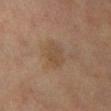<record>
<lighting>cross-polarized</lighting>
<site>right lower leg</site>
<lesion_size>
  <long_diameter_mm_approx>3.0</long_diameter_mm_approx>
</lesion_size>
<patient>
  <sex>female</sex>
  <age_approx>70</age_approx>
</patient>
<image>
  <source>total-body photography crop</source>
  <field_of_view_mm>15</field_of_view_mm>
</image>
</record>